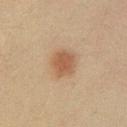  biopsy_status: not biopsied; imaged during a skin examination